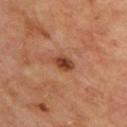<tbp_lesion>
  <biopsy_status>not biopsied; imaged during a skin examination</biopsy_status>
  <site>upper back</site>
  <image>
    <source>total-body photography crop</source>
    <field_of_view_mm>15</field_of_view_mm>
  </image>
  <patient>
    <sex>male</sex>
    <age_approx>65</age_approx>
  </patient>
  <lesion_size>
    <long_diameter_mm_approx>2.5</long_diameter_mm_approx>
  </lesion_size>
</tbp_lesion>